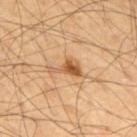Acquisition and patient details: A 15 mm crop from a total-body photograph taken for skin-cancer surveillance. The patient is a male aged around 55. On the upper back. This is a cross-polarized tile.